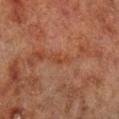<tbp_lesion>
<biopsy_status>not biopsied; imaged during a skin examination</biopsy_status>
<site>right lower leg</site>
<lighting>cross-polarized</lighting>
<lesion_size>
  <long_diameter_mm_approx>3.0</long_diameter_mm_approx>
</lesion_size>
<patient>
  <sex>male</sex>
  <age_approx>70</age_approx>
</patient>
<automated_metrics>
  <area_mm2_approx>2.5</area_mm2_approx>
  <eccentricity>0.9</eccentricity>
  <shape_asymmetry>0.55</shape_asymmetry>
  <cielab_L>36</cielab_L>
  <cielab_a>22</cielab_a>
  <cielab_b>28</cielab_b>
  <vs_skin_darker_L>5.0</vs_skin_darker_L>
  <vs_skin_contrast_norm>5.5</vs_skin_contrast_norm>
  <nevus_likeness_0_100>0</nevus_likeness_0_100>
  <lesion_detection_confidence_0_100>95</lesion_detection_confidence_0_100>
</automated_metrics>
<image>
  <source>total-body photography crop</source>
  <field_of_view_mm>15</field_of_view_mm>
</image>
</tbp_lesion>